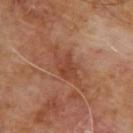Findings:
- follow-up: no biopsy performed (imaged during a skin exam)
- patient: male, approximately 65 years of age
- acquisition: total-body-photography crop, ~15 mm field of view
- lesion diameter: ≈5.5 mm
- body site: the upper back
- illumination: cross-polarized